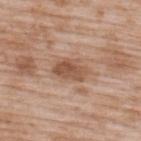No biopsy was performed on this lesion — it was imaged during a full skin examination and was not determined to be concerning. Measured at roughly 4 mm in maximum diameter. A roughly 15 mm field-of-view crop from a total-body skin photograph. A male subject aged around 50. The lesion is on the upper back. The lesion-visualizer software estimated a lesion area of about 7 mm² and an outline eccentricity of about 0.8 (0 = round, 1 = elongated). And it measured a border-irregularity index near 2.5/10 and radial color variation of about 1.5. The software also gave lesion-presence confidence of about 100/100.